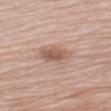Captured during whole-body skin photography for melanoma surveillance; the lesion was not biopsied.
Measured at roughly 4.5 mm in maximum diameter.
An algorithmic analysis of the crop reported a lesion color around L≈60 a*≈18 b*≈27 in CIELAB. The software also gave a within-lesion color-variation index near 5.5/10.
A male patient aged 73–77.
Located on the left upper arm.
A lesion tile, about 15 mm wide, cut from a 3D total-body photograph.